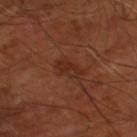The lesion was tiled from a total-body skin photograph and was not biopsied.
A subject roughly 65 years of age.
The lesion's longest dimension is about 3.5 mm.
A roughly 15 mm field-of-view crop from a total-body skin photograph.
On the left thigh.
Automated image analysis of the tile measured a mean CIELAB color near L≈28 a*≈23 b*≈27 and about 6 CIELAB-L* units darker than the surrounding skin. The analysis additionally found internal color variation of about 3 on a 0–10 scale.